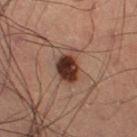Recorded during total-body skin imaging; not selected for excision or biopsy. The subject is a male aged 58–62. A 15 mm close-up tile from a total-body photography series done for melanoma screening. The tile uses cross-polarized illumination. About 4 mm across.